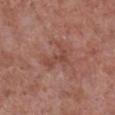<record>
<biopsy_status>not biopsied; imaged during a skin examination</biopsy_status>
<image>
  <source>total-body photography crop</source>
  <field_of_view_mm>15</field_of_view_mm>
</image>
<patient>
  <sex>male</sex>
  <age_approx>55</age_approx>
</patient>
<site>right lower leg</site>
</record>